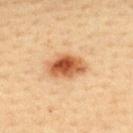Impression:
The lesion was tiled from a total-body skin photograph and was not biopsied.
Context:
The lesion is located on the upper back. The recorded lesion diameter is about 5 mm. The patient is a female roughly 40 years of age. An algorithmic analysis of the crop reported a footprint of about 11 mm². It also reported border irregularity of about 2 on a 0–10 scale. A 15 mm close-up tile from a total-body photography series done for melanoma screening.